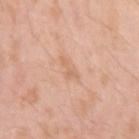Q: What is the imaging modality?
A: 15 mm crop, total-body photography
Q: Lesion size?
A: ~3 mm (longest diameter)
Q: Who is the patient?
A: male, aged around 25
Q: Where on the body is the lesion?
A: the left upper arm